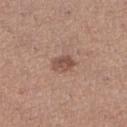workup = imaged on a skin check; not biopsied | location = the right lower leg | subject = female, aged 38–42 | image = total-body-photography crop, ~15 mm field of view | lesion diameter = about 3 mm.